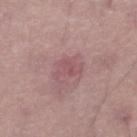Captured during whole-body skin photography for melanoma surveillance; the lesion was not biopsied.
About 3.5 mm across.
This image is a 15 mm lesion crop taken from a total-body photograph.
A male patient, aged approximately 45.
The lesion is on the right lower leg.